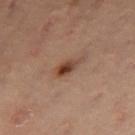Q: Is there a histopathology result?
A: no biopsy performed (imaged during a skin exam)
Q: Who is the patient?
A: female, aged around 55
Q: What is the lesion's diameter?
A: about 3.5 mm
Q: What is the imaging modality?
A: ~15 mm crop, total-body skin-cancer survey
Q: How was the tile lit?
A: cross-polarized illumination
Q: Where on the body is the lesion?
A: the abdomen